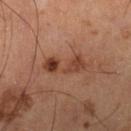This lesion was catalogued during total-body skin photography and was not selected for biopsy. This image is a 15 mm lesion crop taken from a total-body photograph. Automated image analysis of the tile measured a footprint of about 8.5 mm², an outline eccentricity of about 0.95 (0 = round, 1 = elongated), and a shape-asymmetry score of about 0.4 (0 = symmetric). It also reported a border-irregularity index near 6/10, a within-lesion color-variation index near 7.5/10, and radial color variation of about 3. A male patient about 60 years old. From the right lower leg.